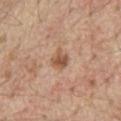body site: the abdomen | lighting: white-light | image source: ~15 mm crop, total-body skin-cancer survey | patient: male, aged around 70 | size: ~2.5 mm (longest diameter) | automated metrics: an average lesion color of about L≈55 a*≈20 b*≈33 (CIELAB), about 11 CIELAB-L* units darker than the surrounding skin, and a normalized border contrast of about 8; a border-irregularity index near 2.5/10 and internal color variation of about 3 on a 0–10 scale.